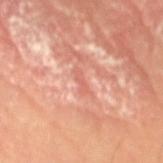notes — catalogued during a skin exam; not biopsied
image-analysis metrics — an outline eccentricity of about 0.95 (0 = round, 1 = elongated) and a shape-asymmetry score of about 0.5 (0 = symmetric); a lesion color around L≈61 a*≈29 b*≈31 in CIELAB, about 7 CIELAB-L* units darker than the surrounding skin, and a normalized lesion–skin contrast near 4.5; a border-irregularity index near 5.5/10 and peripheral color asymmetry of about 0; a classifier nevus-likeness of about 0/100 and a detector confidence of about 60 out of 100 that the crop contains a lesion
patient — male, aged 38–42
image — 15 mm crop, total-body photography
lighting — cross-polarized illumination
diameter — ~2.5 mm (longest diameter)
location — the right upper arm